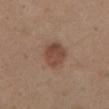The patient is a female approximately 40 years of age. Located on the front of the torso. Longest diameter approximately 3 mm. A lesion tile, about 15 mm wide, cut from a 3D total-body photograph. Automated image analysis of the tile measured a border-irregularity rating of about 1/10 and radial color variation of about 1. The software also gave a classifier nevus-likeness of about 90/100 and lesion-presence confidence of about 100/100. The tile uses white-light illumination.